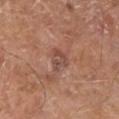A male subject, aged approximately 65.
Cropped from a total-body skin-imaging series; the visible field is about 15 mm.
On the left lower leg.
The tile uses white-light illumination.
The total-body-photography lesion software estimated border irregularity of about 4 on a 0–10 scale and radial color variation of about 1. And it measured a classifier nevus-likeness of about 0/100.